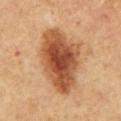Captured during whole-body skin photography for melanoma surveillance; the lesion was not biopsied.
A male subject, aged 58–62.
The lesion is on the mid back.
Cropped from a whole-body photographic skin survey; the tile spans about 15 mm.
An algorithmic analysis of the crop reported a footprint of about 31 mm² and two-axis asymmetry of about 0.2.
Captured under cross-polarized illumination.